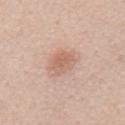The lesion was photographed on a routine skin check and not biopsied; there is no pathology result.
Longest diameter approximately 3 mm.
This image is a 15 mm lesion crop taken from a total-body photograph.
The lesion-visualizer software estimated roughly 9 lightness units darker than nearby skin and a lesion-to-skin contrast of about 6 (normalized; higher = more distinct). The software also gave a border-irregularity index near 2/10 and peripheral color asymmetry of about 1. The software also gave an automated nevus-likeness rating near 55 out of 100 and a detector confidence of about 100 out of 100 that the crop contains a lesion.
A male patient, in their mid- to late 50s.
Located on the mid back.
Captured under white-light illumination.